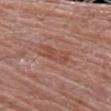Q: Was this lesion biopsied?
A: imaged on a skin check; not biopsied
Q: Where on the body is the lesion?
A: the left upper arm
Q: What is the imaging modality?
A: ~15 mm tile from a whole-body skin photo
Q: Lesion size?
A: about 3.5 mm
Q: What are the patient's age and sex?
A: male, aged around 80
Q: Illumination type?
A: white-light
Q: Automated lesion metrics?
A: a lesion area of about 6 mm² and a symmetry-axis asymmetry near 0.3; an average lesion color of about L≈49 a*≈24 b*≈28 (CIELAB), a lesion–skin lightness drop of about 7, and a normalized border contrast of about 5.5; a nevus-likeness score of about 0/100